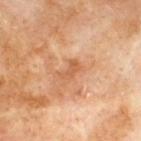biopsy status=catalogued during a skin exam; not biopsied | body site=the upper back | lighting=cross-polarized | acquisition=~15 mm tile from a whole-body skin photo | lesion diameter=about 2.5 mm | patient=male, about 70 years old.